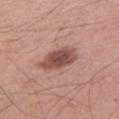Part of a total-body skin-imaging series; this lesion was reviewed on a skin check and was not flagged for biopsy.
A male patient, roughly 50 years of age.
A roughly 15 mm field-of-view crop from a total-body skin photograph.
From the left lower leg.
About 5 mm across.